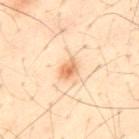{"biopsy_status": "not biopsied; imaged during a skin examination", "site": "upper back", "patient": {"sex": "male", "age_approx": 50}, "automated_metrics": {"area_mm2_approx": 4.0, "eccentricity": 0.75, "cielab_L": 64, "cielab_a": 21, "cielab_b": 36, "vs_skin_contrast_norm": 7.5, "border_irregularity_0_10": 2.5, "color_variation_0_10": 4.0, "peripheral_color_asymmetry": 1.5, "nevus_likeness_0_100": 75, "lesion_detection_confidence_0_100": 100}, "lighting": "cross-polarized", "image": {"source": "total-body photography crop", "field_of_view_mm": 15}}